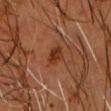The lesion-visualizer software estimated a footprint of about 3.5 mm² and two-axis asymmetry of about 0.2. It also reported an average lesion color of about L≈28 a*≈23 b*≈29 (CIELAB) and a normalized border contrast of about 8.5. The analysis additionally found a border-irregularity index near 2/10 and peripheral color asymmetry of about 0.5. It also reported a classifier nevus-likeness of about 75/100 and lesion-presence confidence of about 100/100. A lesion tile, about 15 mm wide, cut from a 3D total-body photograph. A male patient in their 60s. Longest diameter approximately 2.5 mm. The lesion is located on the head or neck.